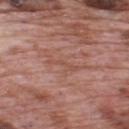| key | value |
|---|---|
| biopsy status | imaged on a skin check; not biopsied |
| automated metrics | an eccentricity of roughly 0.95 and two-axis asymmetry of about 0.65; an average lesion color of about L≈51 a*≈24 b*≈28 (CIELAB), roughly 6 lightness units darker than nearby skin, and a lesion-to-skin contrast of about 5 (normalized; higher = more distinct); an automated nevus-likeness rating near 0 out of 100 and lesion-presence confidence of about 80/100 |
| patient | male, approximately 70 years of age |
| location | the upper back |
| lesion size | ~3 mm (longest diameter) |
| image | total-body-photography crop, ~15 mm field of view |
| illumination | white-light illumination |